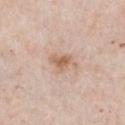Clinical impression: The lesion was tiled from a total-body skin photograph and was not biopsied. Background: The subject is a female aged approximately 45. From the chest. A 15 mm close-up extracted from a 3D total-body photography capture. An algorithmic analysis of the crop reported an area of roughly 5 mm², a shape eccentricity near 0.7, and a symmetry-axis asymmetry near 0.5. Longest diameter approximately 3.5 mm. Imaged with white-light lighting.